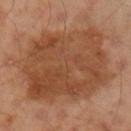The lesion was tiled from a total-body skin photograph and was not biopsied.
The lesion is located on the left upper arm.
A male patient aged 43 to 47.
A 15 mm close-up tile from a total-body photography series done for melanoma screening.